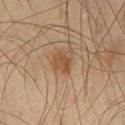No biopsy was performed on this lesion — it was imaged during a full skin examination and was not determined to be concerning. Captured under cross-polarized illumination. The lesion's longest dimension is about 3 mm. The lesion is on the leg. A 15 mm close-up extracted from a 3D total-body photography capture. A male patient, aged 43–47. The lesion-visualizer software estimated a mean CIELAB color near L≈39 a*≈16 b*≈29 and about 7 CIELAB-L* units darker than the surrounding skin. The analysis additionally found a nevus-likeness score of about 60/100 and a lesion-detection confidence of about 100/100.